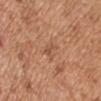biopsy status=total-body-photography surveillance lesion; no biopsy | body site=the right upper arm | automated lesion analysis=a nevus-likeness score of about 0/100 | image=15 mm crop, total-body photography | lesion diameter=about 2.5 mm | illumination=white-light | subject=male, aged 53–57.